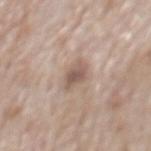{
  "biopsy_status": "not biopsied; imaged during a skin examination",
  "patient": {
    "sex": "male",
    "age_approx": 70
  },
  "site": "mid back",
  "lighting": "white-light",
  "lesion_size": {
    "long_diameter_mm_approx": 3.0
  },
  "automated_metrics": {
    "area_mm2_approx": 5.0,
    "shape_asymmetry": 0.25,
    "border_irregularity_0_10": 2.5,
    "color_variation_0_10": 3.0,
    "peripheral_color_asymmetry": 1.0,
    "lesion_detection_confidence_0_100": 100
  },
  "image": {
    "source": "total-body photography crop",
    "field_of_view_mm": 15
  }
}